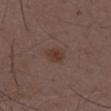biopsy_status: not biopsied; imaged during a skin examination
site: mid back
patient:
  sex: male
  age_approx: 50
image:
  source: total-body photography crop
  field_of_view_mm: 15
automated_metrics:
  border_irregularity_0_10: 2.0
  color_variation_0_10: 2.0
  nevus_likeness_0_100: 65
  lesion_detection_confidence_0_100: 100
lighting: white-light